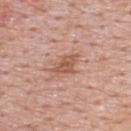Imaged during a routine full-body skin examination; the lesion was not biopsied and no histopathology is available. About 3.5 mm across. From the back. A male subject, in their mid- to late 50s. A close-up tile cropped from a whole-body skin photograph, about 15 mm across.